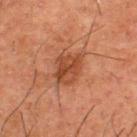Background:
The lesion is located on the upper back. A male patient, in their 50s. A lesion tile, about 15 mm wide, cut from a 3D total-body photograph. About 3.5 mm across. The tile uses cross-polarized illumination.